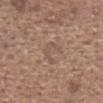biopsy_status: not biopsied; imaged during a skin examination
lesion_size:
  long_diameter_mm_approx: 3.5
site: head or neck
image:
  source: total-body photography crop
  field_of_view_mm: 15
patient:
  sex: male
  age_approx: 65